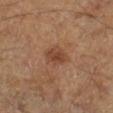{
  "biopsy_status": "not biopsied; imaged during a skin examination",
  "lighting": "cross-polarized",
  "lesion_size": {
    "long_diameter_mm_approx": 3.0
  },
  "image": {
    "source": "total-body photography crop",
    "field_of_view_mm": 15
  },
  "site": "right lower leg",
  "patient": {
    "sex": "male",
    "age_approx": 60
  }
}